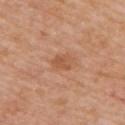Image and clinical context: A 15 mm close-up tile from a total-body photography series done for melanoma screening. Captured under white-light illumination. A male patient aged around 75. Measured at roughly 2.5 mm in maximum diameter. The lesion is located on the upper back.